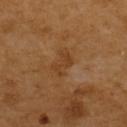{"biopsy_status": "not biopsied; imaged during a skin examination"}